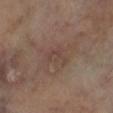notes: no biopsy performed (imaged during a skin exam)
size: ~3 mm (longest diameter)
site: the left lower leg
lighting: cross-polarized
patient: male, approximately 70 years of age
acquisition: ~15 mm tile from a whole-body skin photo
automated metrics: a footprint of about 3.5 mm², a shape eccentricity near 0.8, and two-axis asymmetry of about 0.6; an average lesion color of about L≈40 a*≈16 b*≈21 (CIELAB), about 5 CIELAB-L* units darker than the surrounding skin, and a lesion-to-skin contrast of about 4.5 (normalized; higher = more distinct); a border-irregularity rating of about 8/10, internal color variation of about 0 on a 0–10 scale, and a peripheral color-asymmetry measure near 0; a lesion-detection confidence of about 100/100